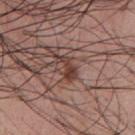No biopsy was performed on this lesion — it was imaged during a full skin examination and was not determined to be concerning.
The subject is a male aged around 55.
The lesion is located on the chest.
The recorded lesion diameter is about 3.5 mm.
Imaged with white-light lighting.
A region of skin cropped from a whole-body photographic capture, roughly 15 mm wide.